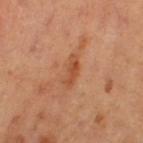Part of a total-body skin-imaging series; this lesion was reviewed on a skin check and was not flagged for biopsy. An algorithmic analysis of the crop reported an area of roughly 4.5 mm² and two-axis asymmetry of about 0.25. The software also gave border irregularity of about 3 on a 0–10 scale. And it measured a nevus-likeness score of about 0/100. A female patient, roughly 60 years of age. A close-up tile cropped from a whole-body skin photograph, about 15 mm across. Imaged with cross-polarized lighting. The lesion's longest dimension is about 4 mm. The lesion is on the left thigh.